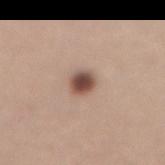Captured during whole-body skin photography for melanoma surveillance; the lesion was not biopsied.
A 15 mm crop from a total-body photograph taken for skin-cancer surveillance.
A female subject, roughly 65 years of age.
On the abdomen.